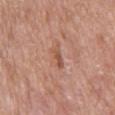Clinical impression:
Imaged during a routine full-body skin examination; the lesion was not biopsied and no histopathology is available.
Context:
Automated image analysis of the tile measured a lesion area of about 3.5 mm², an eccentricity of roughly 0.9, and two-axis asymmetry of about 0.35. The analysis additionally found a border-irregularity index near 4/10, a color-variation rating of about 0.5/10, and radial color variation of about 0. The analysis additionally found a classifier nevus-likeness of about 0/100 and lesion-presence confidence of about 95/100. The subject is a female about 65 years old. Located on the upper back. Longest diameter approximately 3 mm. Cropped from a total-body skin-imaging series; the visible field is about 15 mm.